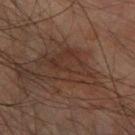Q: Was a biopsy performed?
A: no biopsy performed (imaged during a skin exam)
Q: What is the imaging modality?
A: 15 mm crop, total-body photography
Q: Patient demographics?
A: male, aged around 75
Q: How large is the lesion?
A: about 6.5 mm
Q: What lighting was used for the tile?
A: cross-polarized
Q: Where on the body is the lesion?
A: the right forearm